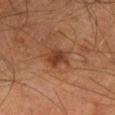Assessment: No biopsy was performed on this lesion — it was imaged during a full skin examination and was not determined to be concerning. Image and clinical context: A male subject aged 63 to 67. From the left thigh. About 3 mm across. A region of skin cropped from a whole-body photographic capture, roughly 15 mm wide. Automated tile analysis of the lesion measured a footprint of about 5.5 mm², an outline eccentricity of about 0.7 (0 = round, 1 = elongated), and a symmetry-axis asymmetry near 0.3. And it measured a lesion color around L≈34 a*≈23 b*≈29 in CIELAB, about 9 CIELAB-L* units darker than the surrounding skin, and a normalized lesion–skin contrast near 8. And it measured a border-irregularity index near 3/10 and internal color variation of about 3 on a 0–10 scale. Captured under cross-polarized illumination.